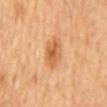This lesion was catalogued during total-body skin photography and was not selected for biopsy.
The lesion-visualizer software estimated a footprint of about 8 mm². It also reported a mean CIELAB color near L≈61 a*≈24 b*≈42 and about 11 CIELAB-L* units darker than the surrounding skin. It also reported a border-irregularity rating of about 2.5/10 and a peripheral color-asymmetry measure near 1.5. It also reported a classifier nevus-likeness of about 75/100 and a lesion-detection confidence of about 100/100.
On the mid back.
A 15 mm close-up extracted from a 3D total-body photography capture.
A male patient aged 63 to 67.